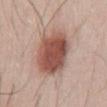workup = no biopsy performed (imaged during a skin exam) | location = the chest | subject = male, approximately 25 years of age | tile lighting = white-light illumination | size = about 6.5 mm | image-analysis metrics = a mean CIELAB color near L≈52 a*≈22 b*≈26 and a lesion–skin lightness drop of about 16; border irregularity of about 1.5 on a 0–10 scale; a classifier nevus-likeness of about 100/100 | image = 15 mm crop, total-body photography.